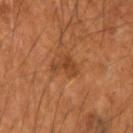follow-up = no biopsy performed (imaged during a skin exam)
image source = ~15 mm tile from a whole-body skin photo
tile lighting = cross-polarized illumination
anatomic site = the left forearm
patient = male, approximately 50 years of age
diameter = about 3 mm